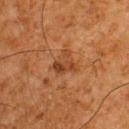Imaged during a routine full-body skin examination; the lesion was not biopsied and no histopathology is available.